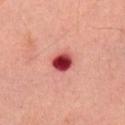Q: Patient demographics?
A: male, approximately 45 years of age
Q: What did automated image analysis measure?
A: an area of roughly 5 mm², an outline eccentricity of about 0.5 (0 = round, 1 = elongated), and two-axis asymmetry of about 0.2
Q: What lighting was used for the tile?
A: cross-polarized
Q: What is the lesion's diameter?
A: ~2.5 mm (longest diameter)
Q: What is the imaging modality?
A: 15 mm crop, total-body photography
Q: What is the anatomic site?
A: the right upper arm